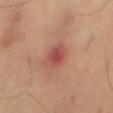Recorded during total-body skin imaging; not selected for excision or biopsy.
The patient is a male aged 43–47.
A region of skin cropped from a whole-body photographic capture, roughly 15 mm wide.
The lesion's longest dimension is about 3.5 mm.
Located on the leg.